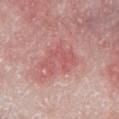Q: Was a biopsy performed?
A: total-body-photography surveillance lesion; no biopsy
Q: Automated lesion metrics?
A: a lesion area of about 10 mm² and an eccentricity of roughly 0.8; an average lesion color of about L≈57 a*≈29 b*≈24 (CIELAB), roughly 7 lightness units darker than nearby skin, and a normalized border contrast of about 5; border irregularity of about 7.5 on a 0–10 scale, a within-lesion color-variation index near 2/10, and peripheral color asymmetry of about 0.5; a nevus-likeness score of about 0/100
Q: How large is the lesion?
A: ≈5 mm
Q: Who is the patient?
A: male, aged around 65
Q: Where on the body is the lesion?
A: the left lower leg
Q: How was this image acquired?
A: 15 mm crop, total-body photography